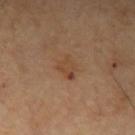Clinical impression: This lesion was catalogued during total-body skin photography and was not selected for biopsy. Clinical summary: A close-up tile cropped from a whole-body skin photograph, about 15 mm across. A male patient, aged 58 to 62. This is a cross-polarized tile. The lesion is located on the right upper arm. The recorded lesion diameter is about 3 mm.